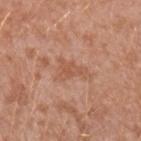  biopsy_status: not biopsied; imaged during a skin examination